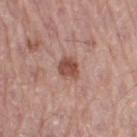notes: total-body-photography surveillance lesion; no biopsy
tile lighting: white-light
location: the right thigh
image: total-body-photography crop, ~15 mm field of view
lesion diameter: about 3 mm
automated lesion analysis: a footprint of about 5.5 mm², a shape eccentricity near 0.55, and a shape-asymmetry score of about 0.15 (0 = symmetric); a mean CIELAB color near L≈50 a*≈23 b*≈26 and a lesion–skin lightness drop of about 12; a classifier nevus-likeness of about 90/100
patient: male, in their mid- to late 50s